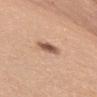<lesion>
  <site>chest</site>
  <patient>
    <sex>female</sex>
    <age_approx>55</age_approx>
  </patient>
  <image>
    <source>total-body photography crop</source>
    <field_of_view_mm>15</field_of_view_mm>
  </image>
  <lesion_size>
    <long_diameter_mm_approx>3.0</long_diameter_mm_approx>
  </lesion_size>
</lesion>